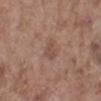From the left lower leg. Captured under white-light illumination. Measured at roughly 2.5 mm in maximum diameter. This image is a 15 mm lesion crop taken from a total-body photograph. The patient is a female aged around 85 or older.